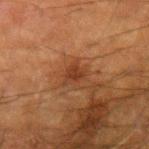A male subject, aged 63–67. A 15 mm close-up tile from a total-body photography series done for melanoma screening. The tile uses cross-polarized illumination. Longest diameter approximately 3 mm. From the left forearm.